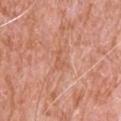The lesion was tiled from a total-body skin photograph and was not biopsied. A 15 mm close-up extracted from a 3D total-body photography capture. The recorded lesion diameter is about 2.5 mm. A male subject, aged approximately 80. Captured under white-light illumination. The lesion is located on the head or neck.